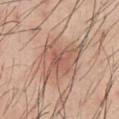Imaged during a routine full-body skin examination; the lesion was not biopsied and no histopathology is available.
The lesion is on the chest.
The tile uses white-light illumination.
A 15 mm close-up tile from a total-body photography series done for melanoma screening.
About 5.5 mm across.
Automated tile analysis of the lesion measured a footprint of about 13 mm², a shape eccentricity near 0.8, and a shape-asymmetry score of about 0.45 (0 = symmetric). It also reported a mean CIELAB color near L≈57 a*≈21 b*≈28, a lesion–skin lightness drop of about 9, and a lesion-to-skin contrast of about 5.5 (normalized; higher = more distinct). And it measured a border-irregularity rating of about 5.5/10, internal color variation of about 4.5 on a 0–10 scale, and peripheral color asymmetry of about 1.
A male patient, aged approximately 45.